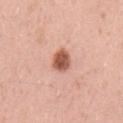Acquisition and patient details: Automated image analysis of the tile measured an average lesion color of about L≈57 a*≈26 b*≈31 (CIELAB), roughly 16 lightness units darker than nearby skin, and a lesion-to-skin contrast of about 10.5 (normalized; higher = more distinct). And it measured a nevus-likeness score of about 100/100 and lesion-presence confidence of about 100/100. A 15 mm crop from a total-body photograph taken for skin-cancer surveillance. A male subject, aged 53 to 57. About 3 mm across. The tile uses white-light illumination. The lesion is located on the chest.